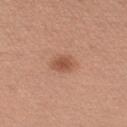notes: imaged on a skin check; not biopsied | lesion diameter: ~3 mm (longest diameter) | anatomic site: the left upper arm | patient: female, about 30 years old | image: ~15 mm tile from a whole-body skin photo | automated metrics: a lesion area of about 5.5 mm², an outline eccentricity of about 0.75 (0 = round, 1 = elongated), and a symmetry-axis asymmetry near 0.2; a mean CIELAB color near L≈53 a*≈24 b*≈31 and a lesion-to-skin contrast of about 7 (normalized; higher = more distinct); a classifier nevus-likeness of about 95/100.